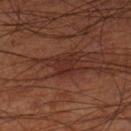Recorded during total-body skin imaging; not selected for excision or biopsy.
A 15 mm close-up extracted from a 3D total-body photography capture.
On the leg.
The subject is a male aged approximately 70.